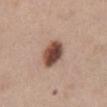Clinical impression: Captured during whole-body skin photography for melanoma surveillance; the lesion was not biopsied. Context: On the abdomen. Cropped from a total-body skin-imaging series; the visible field is about 15 mm. The subject is a female aged around 40.